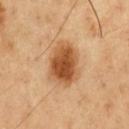biopsy status: catalogued during a skin exam; not biopsied | site: the chest | image source: total-body-photography crop, ~15 mm field of view | lighting: cross-polarized illumination | lesion size: about 5.5 mm | patient: male, roughly 50 years of age.